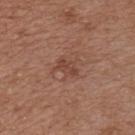| key | value |
|---|---|
| notes | total-body-photography surveillance lesion; no biopsy |
| image-analysis metrics | a lesion area of about 4 mm², an outline eccentricity of about 0.8 (0 = round, 1 = elongated), and a symmetry-axis asymmetry near 0.35; a border-irregularity index near 3.5/10, a color-variation rating of about 1.5/10, and radial color variation of about 0.5 |
| acquisition | ~15 mm crop, total-body skin-cancer survey |
| subject | male, aged around 55 |
| lesion size | ~3 mm (longest diameter) |
| tile lighting | white-light illumination |
| location | the chest |